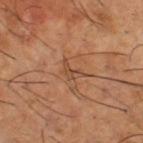Q: Was a biopsy performed?
A: catalogued during a skin exam; not biopsied
Q: Patient demographics?
A: male, about 65 years old
Q: What kind of image is this?
A: total-body-photography crop, ~15 mm field of view
Q: What is the anatomic site?
A: the right thigh